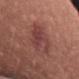The lesion was tiled from a total-body skin photograph and was not biopsied. A 15 mm close-up extracted from a 3D total-body photography capture. On the right thigh. A female subject aged approximately 55. The lesion-visualizer software estimated a footprint of about 12 mm², an eccentricity of roughly 0.85, and a shape-asymmetry score of about 0.5 (0 = symmetric). The analysis additionally found about 7 CIELAB-L* units darker than the surrounding skin and a lesion-to-skin contrast of about 6.5 (normalized; higher = more distinct). This is a white-light tile.